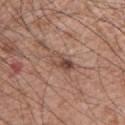Captured during whole-body skin photography for melanoma surveillance; the lesion was not biopsied.